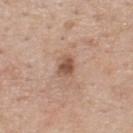Q: Was a biopsy performed?
A: imaged on a skin check; not biopsied
Q: Patient demographics?
A: male, approximately 60 years of age
Q: What is the imaging modality?
A: ~15 mm tile from a whole-body skin photo
Q: Where on the body is the lesion?
A: the upper back
Q: Lesion size?
A: ~2.5 mm (longest diameter)